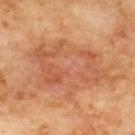Impression: Part of a total-body skin-imaging series; this lesion was reviewed on a skin check and was not flagged for biopsy. Background: Cropped from a total-body skin-imaging series; the visible field is about 15 mm. The lesion is on the upper back. Measured at roughly 10.5 mm in maximum diameter. Automated tile analysis of the lesion measured a mean CIELAB color near L≈59 a*≈27 b*≈37. It also reported a within-lesion color-variation index near 5.5/10 and a peripheral color-asymmetry measure near 1.5. Captured under cross-polarized illumination.